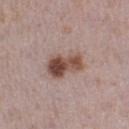{
  "biopsy_status": "not biopsied; imaged during a skin examination",
  "site": "right lower leg",
  "image": {
    "source": "total-body photography crop",
    "field_of_view_mm": 15
  },
  "patient": {
    "sex": "female",
    "age_approx": 30
  },
  "lighting": "white-light"
}